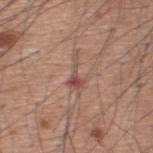Acquisition and patient details:
The patient is a male aged 58–62. Automated image analysis of the tile measured a mean CIELAB color near L≈50 a*≈21 b*≈25, about 9 CIELAB-L* units darker than the surrounding skin, and a normalized border contrast of about 7. The software also gave a border-irregularity rating of about 8.5/10 and a peripheral color-asymmetry measure near 0.5. This is a white-light tile. The lesion is located on the upper back. About 4 mm across. A region of skin cropped from a whole-body photographic capture, roughly 15 mm wide.